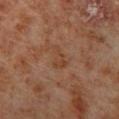Assessment: Imaged during a routine full-body skin examination; the lesion was not biopsied and no histopathology is available. Image and clinical context: Automated tile analysis of the lesion measured border irregularity of about 5 on a 0–10 scale and a within-lesion color-variation index near 1.5/10. It also reported a classifier nevus-likeness of about 0/100. A region of skin cropped from a whole-body photographic capture, roughly 15 mm wide. Measured at roughly 3 mm in maximum diameter. The lesion is located on the right lower leg. The tile uses cross-polarized illumination. A male subject aged around 70.